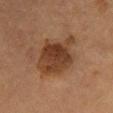Q: Was this lesion biopsied?
A: no biopsy performed (imaged during a skin exam)
Q: Patient demographics?
A: male, aged 53–57
Q: What is the imaging modality?
A: ~15 mm crop, total-body skin-cancer survey
Q: How large is the lesion?
A: ≈6 mm
Q: What is the anatomic site?
A: the mid back
Q: Automated lesion metrics?
A: internal color variation of about 4.5 on a 0–10 scale and peripheral color asymmetry of about 1.5; a detector confidence of about 100 out of 100 that the crop contains a lesion